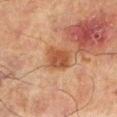{
  "patient": {
    "sex": "male",
    "age_approx": 65
  },
  "image": {
    "source": "total-body photography crop",
    "field_of_view_mm": 15
  },
  "automated_metrics": {
    "color_variation_0_10": 3.0,
    "peripheral_color_asymmetry": 1.0
  },
  "lighting": "cross-polarized",
  "site": "right lower leg",
  "lesion_size": {
    "long_diameter_mm_approx": 3.5
  }
}